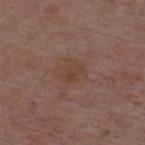Findings:
- workup — no biopsy performed (imaged during a skin exam)